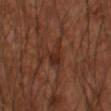follow-up: no biopsy performed (imaged during a skin exam).